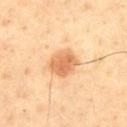This lesion was catalogued during total-body skin photography and was not selected for biopsy. Approximately 3.5 mm at its widest. A male subject in their 60s. The total-body-photography lesion software estimated a lesion–skin lightness drop of about 12. It also reported a border-irregularity rating of about 1.5/10, internal color variation of about 3 on a 0–10 scale, and radial color variation of about 1. The analysis additionally found an automated nevus-likeness rating near 100 out of 100 and a detector confidence of about 100 out of 100 that the crop contains a lesion. The lesion is located on the chest. Imaged with cross-polarized lighting. A roughly 15 mm field-of-view crop from a total-body skin photograph.